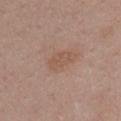– follow-up: no biopsy performed (imaged during a skin exam)
– patient: female, aged 53–57
– image source: 15 mm crop, total-body photography
– body site: the chest